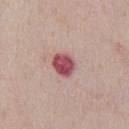Findings:
• image: total-body-photography crop, ~15 mm field of view
• location: the chest
• patient: male, aged 38–42